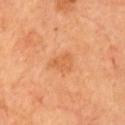Q: Was a biopsy performed?
A: total-body-photography surveillance lesion; no biopsy
Q: What is the imaging modality?
A: ~15 mm tile from a whole-body skin photo
Q: What is the lesion's diameter?
A: ≈3 mm
Q: Lesion location?
A: the chest
Q: Patient demographics?
A: male, aged 68–72